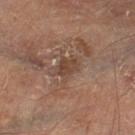Q: Was this lesion biopsied?
A: total-body-photography surveillance lesion; no biopsy
Q: Who is the patient?
A: male, approximately 70 years of age
Q: What lighting was used for the tile?
A: cross-polarized
Q: What kind of image is this?
A: total-body-photography crop, ~15 mm field of view
Q: Lesion size?
A: ~2.5 mm (longest diameter)
Q: Lesion location?
A: the left lower leg